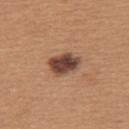Q: Was this lesion biopsied?
A: no biopsy performed (imaged during a skin exam)
Q: What kind of image is this?
A: 15 mm crop, total-body photography
Q: How large is the lesion?
A: ≈4 mm
Q: How was the tile lit?
A: white-light illumination
Q: Patient demographics?
A: female, aged around 30
Q: Lesion location?
A: the upper back